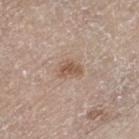The lesion was photographed on a routine skin check and not biopsied; there is no pathology result. About 2.5 mm across. The lesion-visualizer software estimated a footprint of about 4.5 mm², an eccentricity of roughly 0.75, and a shape-asymmetry score of about 0.4 (0 = symmetric). The software also gave a classifier nevus-likeness of about 35/100. From the left thigh. This is a white-light tile. A male patient aged 78–82. A 15 mm crop from a total-body photograph taken for skin-cancer surveillance.